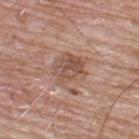Assessment:
Imaged during a routine full-body skin examination; the lesion was not biopsied and no histopathology is available.
Clinical summary:
A close-up tile cropped from a whole-body skin photograph, about 15 mm across. Located on the upper back. A male subject aged around 65.